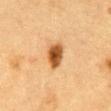Part of a total-body skin-imaging series; this lesion was reviewed on a skin check and was not flagged for biopsy. The lesion is on the abdomen. Cropped from a whole-body photographic skin survey; the tile spans about 15 mm. A male subject, in their mid-80s or older.